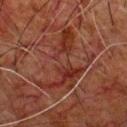{
  "biopsy_status": "not biopsied; imaged during a skin examination",
  "lighting": "cross-polarized",
  "patient": {
    "sex": "male",
    "age_approx": 80
  },
  "site": "chest",
  "image": {
    "source": "total-body photography crop",
    "field_of_view_mm": 15
  }
}